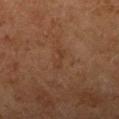{
  "patient": {
    "sex": "female",
    "age_approx": 60
  },
  "image": {
    "source": "total-body photography crop",
    "field_of_view_mm": 15
  },
  "site": "arm"
}